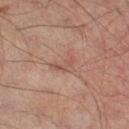Q: Was a biopsy performed?
A: imaged on a skin check; not biopsied
Q: What is the anatomic site?
A: the leg
Q: What is the lesion's diameter?
A: ≈3 mm
Q: How was the tile lit?
A: cross-polarized
Q: Automated lesion metrics?
A: a lesion area of about 4.5 mm², an eccentricity of roughly 0.75, and a symmetry-axis asymmetry near 0.65; a mean CIELAB color near L≈50 a*≈20 b*≈26 and roughly 7 lightness units darker than nearby skin
Q: How was this image acquired?
A: 15 mm crop, total-body photography
Q: Who is the patient?
A: male, roughly 65 years of age